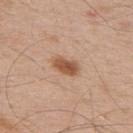Part of a total-body skin-imaging series; this lesion was reviewed on a skin check and was not flagged for biopsy.
Automated tile analysis of the lesion measured an area of roughly 6 mm² and two-axis asymmetry of about 0.2. And it measured a lesion–skin lightness drop of about 12 and a normalized border contrast of about 8.5. And it measured a border-irregularity rating of about 2/10, a within-lesion color-variation index near 3.5/10, and a peripheral color-asymmetry measure near 1.5.
The subject is a male in their 50s.
From the upper back.
Cropped from a whole-body photographic skin survey; the tile spans about 15 mm.
The recorded lesion diameter is about 3.5 mm.